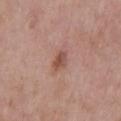Impression: The lesion was tiled from a total-body skin photograph and was not biopsied. Acquisition and patient details: The lesion-visualizer software estimated an area of roughly 3.5 mm² and two-axis asymmetry of about 0.25. The software also gave an average lesion color of about L≈51 a*≈22 b*≈27 (CIELAB), about 10 CIELAB-L* units darker than the surrounding skin, and a normalized lesion–skin contrast near 7.5. A male patient aged 58 to 62. About 3 mm across. The tile uses white-light illumination. On the chest. Cropped from a whole-body photographic skin survey; the tile spans about 15 mm.